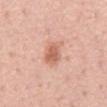Captured during whole-body skin photography for melanoma surveillance; the lesion was not biopsied.